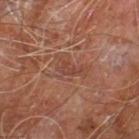Recorded during total-body skin imaging; not selected for excision or biopsy. A roughly 15 mm field-of-view crop from a total-body skin photograph. About 2.5 mm across. A male subject approximately 60 years of age. Imaged with cross-polarized lighting. From the right leg. The lesion-visualizer software estimated a lesion area of about 2.5 mm², an eccentricity of roughly 0.9, and two-axis asymmetry of about 0.45. The analysis additionally found a lesion–skin lightness drop of about 6 and a normalized lesion–skin contrast near 5.5.